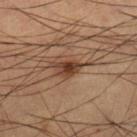This lesion was catalogued during total-body skin photography and was not selected for biopsy.